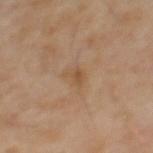Notes:
- follow-up · imaged on a skin check; not biopsied
- acquisition · ~15 mm tile from a whole-body skin photo
- image-analysis metrics · a footprint of about 3 mm², a shape eccentricity near 0.8, and a symmetry-axis asymmetry near 0.55; a mean CIELAB color near L≈49 a*≈17 b*≈33; border irregularity of about 5 on a 0–10 scale, a color-variation rating of about 1.5/10, and a peripheral color-asymmetry measure near 0.5; lesion-presence confidence of about 100/100
- size · ~2.5 mm (longest diameter)
- subject · male, roughly 65 years of age
- tile lighting · cross-polarized